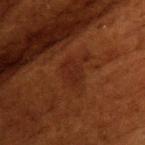subject: female, aged around 80
anatomic site: the chest
imaging modality: ~15 mm tile from a whole-body skin photo